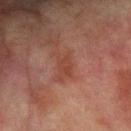biopsy_status: not biopsied; imaged during a skin examination
lighting: cross-polarized
image:
  source: total-body photography crop
  field_of_view_mm: 15
patient:
  sex: male
  age_approx: 75
site: left forearm